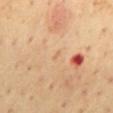<case>
  <biopsy_status>not biopsied; imaged during a skin examination</biopsy_status>
  <automated_metrics>
    <area_mm2_approx>1.0</area_mm2_approx>
    <eccentricity>0.85</eccentricity>
  </automated_metrics>
  <lesion_size>
    <long_diameter_mm_approx>1.5</long_diameter_mm_approx>
  </lesion_size>
  <site>mid back</site>
  <patient>
    <sex>male</sex>
    <age_approx>55</age_approx>
  </patient>
  <lighting>cross-polarized</lighting>
  <image>
    <source>total-body photography crop</source>
    <field_of_view_mm>15</field_of_view_mm>
  </image>
</case>